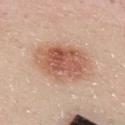The lesion was photographed on a routine skin check and not biopsied; there is no pathology result. About 7 mm across. The subject is a male aged approximately 25. A lesion tile, about 15 mm wide, cut from a 3D total-body photograph. On the upper back. Captured under white-light illumination.